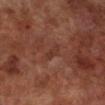Part of a total-body skin-imaging series; this lesion was reviewed on a skin check and was not flagged for biopsy. From the right lower leg. The recorded lesion diameter is about 2.5 mm. Captured under cross-polarized illumination. A male patient in their 70s. This image is a 15 mm lesion crop taken from a total-body photograph.